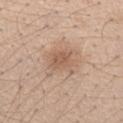Case summary:
– workup — total-body-photography surveillance lesion; no biopsy
– location — the left forearm
– lesion size — about 3.5 mm
– image — ~15 mm crop, total-body skin-cancer survey
– subject — female, approximately 40 years of age
– tile lighting — white-light